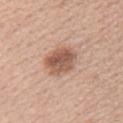Clinical impression: Captured during whole-body skin photography for melanoma surveillance; the lesion was not biopsied. Image and clinical context: Imaged with white-light lighting. The patient is a female approximately 40 years of age. The lesion is on the upper back. The lesion's longest dimension is about 4 mm. A 15 mm close-up tile from a total-body photography series done for melanoma screening.